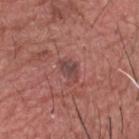Impression:
Part of a total-body skin-imaging series; this lesion was reviewed on a skin check and was not flagged for biopsy.
Context:
The lesion is on the head or neck. The recorded lesion diameter is about 3 mm. A close-up tile cropped from a whole-body skin photograph, about 15 mm across. The patient is a male aged approximately 75. The total-body-photography lesion software estimated a lesion area of about 4 mm², an outline eccentricity of about 0.75 (0 = round, 1 = elongated), and a shape-asymmetry score of about 0.3 (0 = symmetric). It also reported about 8 CIELAB-L* units darker than the surrounding skin and a normalized lesion–skin contrast near 7. The software also gave border irregularity of about 3 on a 0–10 scale and peripheral color asymmetry of about 0.5.